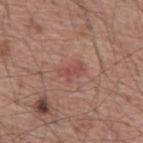Findings:
- image — 15 mm crop, total-body photography
- subject — male, approximately 55 years of age
- lesion diameter — about 3 mm
- site — the mid back
- image-analysis metrics — an eccentricity of roughly 0.85 and two-axis asymmetry of about 0.35; a nevus-likeness score of about 0/100 and a detector confidence of about 100 out of 100 that the crop contains a lesion
- illumination — white-light illumination